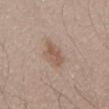{"biopsy_status": "not biopsied; imaged during a skin examination", "automated_metrics": {"vs_skin_darker_L": 8.0, "vs_skin_contrast_norm": 6.5, "border_irregularity_0_10": 2.5, "color_variation_0_10": 2.5, "peripheral_color_asymmetry": 1.0, "nevus_likeness_0_100": 10, "lesion_detection_confidence_0_100": 100}, "image": {"source": "total-body photography crop", "field_of_view_mm": 15}, "site": "lower back", "patient": {"sex": "male", "age_approx": 55}, "lighting": "white-light"}